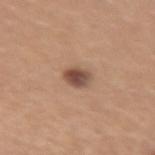Q: Was this lesion biopsied?
A: imaged on a skin check; not biopsied
Q: What is the lesion's diameter?
A: ≈2.5 mm
Q: Patient demographics?
A: female, aged approximately 25
Q: What lighting was used for the tile?
A: white-light
Q: Lesion location?
A: the left forearm
Q: What is the imaging modality?
A: 15 mm crop, total-body photography